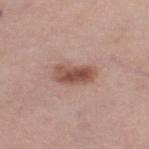Impression: No biopsy was performed on this lesion — it was imaged during a full skin examination and was not determined to be concerning. Acquisition and patient details: The lesion is located on the right lower leg. A 15 mm crop from a total-body photograph taken for skin-cancer surveillance. A female patient about 40 years old.